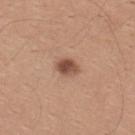• notes: imaged on a skin check; not biopsied
• image-analysis metrics: a shape eccentricity near 0.75 and a symmetry-axis asymmetry near 0.15; a within-lesion color-variation index near 4/10 and peripheral color asymmetry of about 1.5
• location: the mid back
• size: about 3 mm
• image source: ~15 mm tile from a whole-body skin photo
• patient: male, aged 28 to 32
• tile lighting: white-light illumination